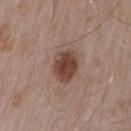The lesion was photographed on a routine skin check and not biopsied; there is no pathology result.
A male subject, in their mid-50s.
The lesion is on the right upper arm.
A roughly 15 mm field-of-view crop from a total-body skin photograph.